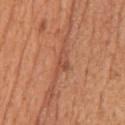Case summary:
- biopsy status: no biopsy performed (imaged during a skin exam)
- tile lighting: white-light
- site: the chest
- diameter: about 3 mm
- imaging modality: total-body-photography crop, ~15 mm field of view
- patient: male, approximately 55 years of age